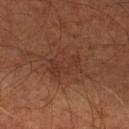Q: Was a biopsy performed?
A: catalogued during a skin exam; not biopsied
Q: Illumination type?
A: cross-polarized illumination
Q: What is the lesion's diameter?
A: ≈4.5 mm
Q: What is the anatomic site?
A: the left lower leg
Q: Who is the patient?
A: approximately 65 years of age
Q: How was this image acquired?
A: total-body-photography crop, ~15 mm field of view